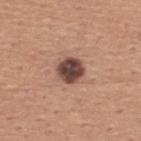{
  "biopsy_status": "not biopsied; imaged during a skin examination",
  "automated_metrics": {
    "cielab_L": 44,
    "cielab_a": 20,
    "cielab_b": 22,
    "vs_skin_darker_L": 19.0,
    "vs_skin_contrast_norm": 14.0,
    "color_variation_0_10": 4.5,
    "peripheral_color_asymmetry": 1.5
  },
  "site": "upper back",
  "lighting": "white-light",
  "image": {
    "source": "total-body photography crop",
    "field_of_view_mm": 15
  },
  "lesion_size": {
    "long_diameter_mm_approx": 3.0
  },
  "patient": {
    "sex": "male",
    "age_approx": 45
  }
}